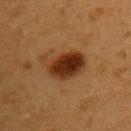Image and clinical context: The lesion is on the upper back. The subject is a male in their mid-50s. This image is a 15 mm lesion crop taken from a total-body photograph.